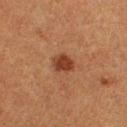  biopsy_status: not biopsied; imaged during a skin examination
  lesion_size:
    long_diameter_mm_approx: 2.5
  image:
    source: total-body photography crop
    field_of_view_mm: 15
  lighting: cross-polarized
  patient:
    sex: female
    age_approx: 40
  site: right thigh